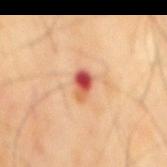Imaged during a routine full-body skin examination; the lesion was not biopsied and no histopathology is available.
A 15 mm close-up extracted from a 3D total-body photography capture.
The recorded lesion diameter is about 3 mm.
The subject is a male aged 68–72.
An algorithmic analysis of the crop reported a footprint of about 4.5 mm², an eccentricity of roughly 0.75, and a shape-asymmetry score of about 0.25 (0 = symmetric). And it measured a nevus-likeness score of about 0/100 and a lesion-detection confidence of about 100/100.
From the mid back.